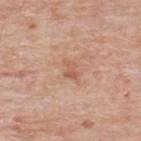{"biopsy_status": "not biopsied; imaged during a skin examination", "lesion_size": {"long_diameter_mm_approx": 2.5}, "site": "upper back", "image": {"source": "total-body photography crop", "field_of_view_mm": 15}, "lighting": "white-light", "patient": {"sex": "male", "age_approx": 75}}